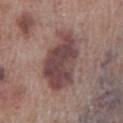Recorded during total-body skin imaging; not selected for excision or biopsy. A male subject aged 68 to 72. An algorithmic analysis of the crop reported an average lesion color of about L≈44 a*≈19 b*≈19 (CIELAB), about 12 CIELAB-L* units darker than the surrounding skin, and a normalized lesion–skin contrast near 9.5. The analysis additionally found border irregularity of about 3 on a 0–10 scale, a within-lesion color-variation index near 4.5/10, and radial color variation of about 1.5. And it measured an automated nevus-likeness rating near 0 out of 100 and a lesion-detection confidence of about 95/100. A 15 mm close-up tile from a total-body photography series done for melanoma screening. Approximately 7.5 mm at its widest. Captured under white-light illumination. On the leg.